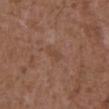Clinical impression:
Recorded during total-body skin imaging; not selected for excision or biopsy.
Image and clinical context:
The recorded lesion diameter is about 2.5 mm. Captured under white-light illumination. From the abdomen. A male subject approximately 75 years of age. A 15 mm close-up tile from a total-body photography series done for melanoma screening. Automated image analysis of the tile measured about 5 CIELAB-L* units darker than the surrounding skin. The analysis additionally found an automated nevus-likeness rating near 0 out of 100 and a detector confidence of about 100 out of 100 that the crop contains a lesion.